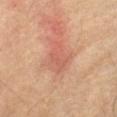Case summary:
- notes · catalogued during a skin exam; not biopsied
- tile lighting · cross-polarized
- subject · male, in their 60s
- body site · the abdomen
- image · ~15 mm tile from a whole-body skin photo
- lesion size · ≈4 mm